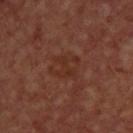The lesion was photographed on a routine skin check and not biopsied; there is no pathology result.
The lesion's longest dimension is about 3.5 mm.
On the upper back.
A female patient, about 45 years old.
Cropped from a total-body skin-imaging series; the visible field is about 15 mm.